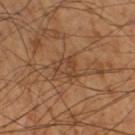notes: imaged on a skin check; not biopsied
illumination: cross-polarized
diameter: ~3 mm (longest diameter)
automated metrics: a shape eccentricity near 0.5 and two-axis asymmetry of about 0.5; border irregularity of about 4.5 on a 0–10 scale, a within-lesion color-variation index near 3.5/10, and peripheral color asymmetry of about 1; a nevus-likeness score of about 0/100 and lesion-presence confidence of about 80/100
acquisition: ~15 mm tile from a whole-body skin photo
anatomic site: the right thigh
patient: male, in their mid-50s